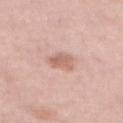{
  "biopsy_status": "not biopsied; imaged during a skin examination",
  "patient": {
    "sex": "female",
    "age_approx": 65
  },
  "lesion_size": {
    "long_diameter_mm_approx": 2.5
  },
  "site": "back",
  "image": {
    "source": "total-body photography crop",
    "field_of_view_mm": 15
  }
}